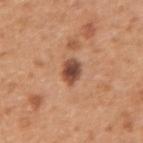No biopsy was performed on this lesion — it was imaged during a full skin examination and was not determined to be concerning.
A male subject aged approximately 55.
Approximately 2.5 mm at its widest.
Cropped from a total-body skin-imaging series; the visible field is about 15 mm.
The lesion is on the left forearm.